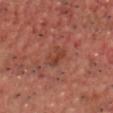No biopsy was performed on this lesion — it was imaged during a full skin examination and was not determined to be concerning. A roughly 15 mm field-of-view crop from a total-body skin photograph. A male subject roughly 50 years of age. Located on the chest.